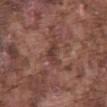{
  "biopsy_status": "not biopsied; imaged during a skin examination",
  "image": {
    "source": "total-body photography crop",
    "field_of_view_mm": 15
  },
  "patient": {
    "sex": "male",
    "age_approx": 75
  },
  "site": "abdomen"
}